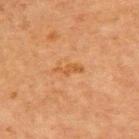The lesion was photographed on a routine skin check and not biopsied; there is no pathology result. A 15 mm close-up tile from a total-body photography series done for melanoma screening. An algorithmic analysis of the crop reported a lesion area of about 3.5 mm², a shape eccentricity near 0.95, and a shape-asymmetry score of about 0.35 (0 = symmetric). The software also gave a nevus-likeness score of about 0/100 and lesion-presence confidence of about 100/100. The patient is a female aged around 55. Measured at roughly 3.5 mm in maximum diameter. From the upper back.